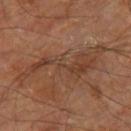Captured during whole-body skin photography for melanoma surveillance; the lesion was not biopsied. The lesion is located on the leg. A lesion tile, about 15 mm wide, cut from a 3D total-body photograph. Captured under cross-polarized illumination. Automated image analysis of the tile measured a footprint of about 22 mm², a shape eccentricity near 0.95, and a symmetry-axis asymmetry near 0.6. The analysis additionally found a border-irregularity index near 9.5/10. Longest diameter approximately 10.5 mm. A male patient, approximately 60 years of age.